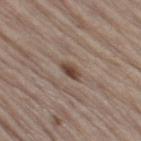<record>
  <site>right thigh</site>
  <image>
    <source>total-body photography crop</source>
    <field_of_view_mm>15</field_of_view_mm>
  </image>
  <patient>
    <sex>male</sex>
    <age_approx>70</age_approx>
  </patient>
</record>